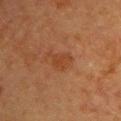Q: Was a biopsy performed?
A: total-body-photography surveillance lesion; no biopsy
Q: Patient demographics?
A: female, aged around 55
Q: What is the anatomic site?
A: the upper back
Q: Automated lesion metrics?
A: a symmetry-axis asymmetry near 0.4
Q: What is the imaging modality?
A: 15 mm crop, total-body photography